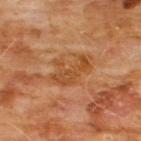workup: no biopsy performed (imaged during a skin exam); location: the chest; illumination: cross-polarized; image: 15 mm crop, total-body photography; patient: male, aged 58 to 62.